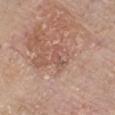biopsy status = catalogued during a skin exam; not biopsied | subject = male, approximately 80 years of age | size = about 2.5 mm | automated lesion analysis = an area of roughly 2.5 mm², a shape eccentricity near 0.85, and a symmetry-axis asymmetry near 0.55; border irregularity of about 6 on a 0–10 scale and peripheral color asymmetry of about 0; an automated nevus-likeness rating near 0 out of 100 and a detector confidence of about 95 out of 100 that the crop contains a lesion | acquisition = ~15 mm tile from a whole-body skin photo | site = the front of the torso | tile lighting = white-light.